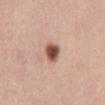Part of a total-body skin-imaging series; this lesion was reviewed on a skin check and was not flagged for biopsy. Cropped from a whole-body photographic skin survey; the tile spans about 15 mm. On the abdomen. This is a white-light tile. A female subject, in their 50s. An algorithmic analysis of the crop reported a lesion color around L≈53 a*≈22 b*≈27 in CIELAB, a lesion–skin lightness drop of about 18, and a normalized border contrast of about 11.5. The analysis additionally found an automated nevus-likeness rating near 100 out of 100 and a detector confidence of about 100 out of 100 that the crop contains a lesion.